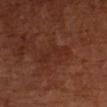Recorded during total-body skin imaging; not selected for excision or biopsy.
A lesion tile, about 15 mm wide, cut from a 3D total-body photograph.
A female subject, aged around 65.
The lesion-visualizer software estimated a mean CIELAB color near L≈27 a*≈23 b*≈27, about 4 CIELAB-L* units darker than the surrounding skin, and a normalized border contrast of about 4.5. And it measured a lesion-detection confidence of about 100/100.
Longest diameter approximately 4.5 mm.
Located on the left upper arm.
This is a cross-polarized tile.